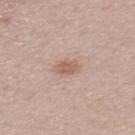The lesion was tiled from a total-body skin photograph and was not biopsied. The subject is a male in their 60s. A 15 mm close-up tile from a total-body photography series done for melanoma screening. The recorded lesion diameter is about 2.5 mm. An algorithmic analysis of the crop reported about 9 CIELAB-L* units darker than the surrounding skin and a normalized lesion–skin contrast near 7. The software also gave a border-irregularity index near 2.5/10, a within-lesion color-variation index near 2/10, and peripheral color asymmetry of about 1. And it measured a classifier nevus-likeness of about 60/100 and a lesion-detection confidence of about 100/100. The lesion is on the upper back.